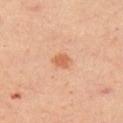biopsy_status: not biopsied; imaged during a skin examination
site: right upper arm
automated_metrics:
  vs_skin_darker_L: 10.0
  border_irregularity_0_10: 2.5
  peripheral_color_asymmetry: 0.5
  nevus_likeness_0_100: 90
  lesion_detection_confidence_0_100: 100
lighting: cross-polarized
patient:
  sex: female
  age_approx: 40
image:
  source: total-body photography crop
  field_of_view_mm: 15
lesion_size:
  long_diameter_mm_approx: 2.5